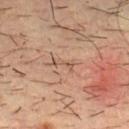Imaged during a routine full-body skin examination; the lesion was not biopsied and no histopathology is available.
Cropped from a whole-body photographic skin survey; the tile spans about 15 mm.
The lesion-visualizer software estimated a footprint of about 2.5 mm², an eccentricity of roughly 0.95, and a shape-asymmetry score of about 0.4 (0 = symmetric). And it measured a lesion color around L≈50 a*≈18 b*≈27 in CIELAB, a lesion–skin lightness drop of about 7, and a normalized border contrast of about 5.5. The analysis additionally found a border-irregularity rating of about 5.5/10, a within-lesion color-variation index near 0/10, and peripheral color asymmetry of about 0.
The lesion's longest dimension is about 3 mm.
A male patient, approximately 35 years of age.
The lesion is on the chest.
This is a cross-polarized tile.